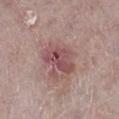Clinical impression: The lesion was photographed on a routine skin check and not biopsied; there is no pathology result. Clinical summary: On the right lower leg. A lesion tile, about 15 mm wide, cut from a 3D total-body photograph. About 4.5 mm across. The patient is a male about 80 years old. Captured under white-light illumination.